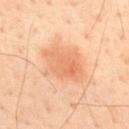Q: Was a biopsy performed?
A: catalogued during a skin exam; not biopsied
Q: Automated lesion metrics?
A: about 9 CIELAB-L* units darker than the surrounding skin
Q: What is the imaging modality?
A: total-body-photography crop, ~15 mm field of view
Q: Lesion location?
A: the mid back
Q: Patient demographics?
A: male, roughly 45 years of age
Q: Illumination type?
A: cross-polarized
Q: How large is the lesion?
A: about 5 mm